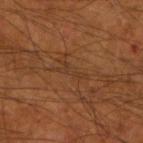notes — imaged on a skin check; not biopsied
imaging modality — ~15 mm crop, total-body skin-cancer survey
subject — male, aged approximately 55
lesion size — ~3.5 mm (longest diameter)
body site — the arm
illumination — cross-polarized
TBP lesion metrics — a nevus-likeness score of about 0/100 and lesion-presence confidence of about 50/100